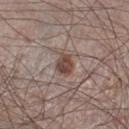No biopsy was performed on this lesion — it was imaged during a full skin examination and was not determined to be concerning. A male subject aged 58–62. Cropped from a total-body skin-imaging series; the visible field is about 15 mm. On the right lower leg. Automated image analysis of the tile measured a border-irregularity index near 1.5/10 and a peripheral color-asymmetry measure near 1. The tile uses white-light illumination.